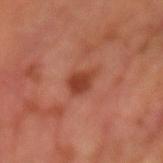Q: Was this lesion biopsied?
A: catalogued during a skin exam; not biopsied
Q: What kind of image is this?
A: 15 mm crop, total-body photography
Q: How was the tile lit?
A: cross-polarized
Q: Patient demographics?
A: male, roughly 65 years of age
Q: What is the lesion's diameter?
A: about 3 mm
Q: Automated lesion metrics?
A: an area of roughly 5 mm², an outline eccentricity of about 0.7 (0 = round, 1 = elongated), and two-axis asymmetry of about 0.25; lesion-presence confidence of about 100/100